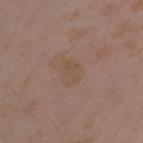subject=female, aged 33 to 37
imaging modality=~15 mm crop, total-body skin-cancer survey
anatomic site=the left upper arm